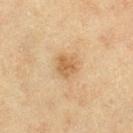workup: no biopsy performed (imaged during a skin exam)
automated metrics: a lesion area of about 5.5 mm², a shape eccentricity near 0.35, and two-axis asymmetry of about 0.2; an automated nevus-likeness rating near 70 out of 100
size: ≈2.5 mm
illumination: cross-polarized illumination
body site: the left thigh
image source: ~15 mm tile from a whole-body skin photo
patient: female, aged approximately 55